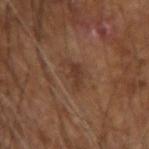Q: How large is the lesion?
A: ~3.5 mm (longest diameter)
Q: Who is the patient?
A: male, aged around 60
Q: What is the imaging modality?
A: 15 mm crop, total-body photography
Q: What is the anatomic site?
A: the arm